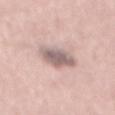Notes:
• notes · total-body-photography surveillance lesion; no biopsy
• location · the mid back
• patient · male, aged approximately 40
• tile lighting · white-light
• acquisition · 15 mm crop, total-body photography
• diameter · ≈3.5 mm